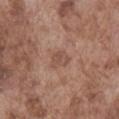Notes:
* follow-up — total-body-photography surveillance lesion; no biopsy
* size — about 2.5 mm
* lighting — white-light illumination
* anatomic site — the abdomen
* imaging modality — 15 mm crop, total-body photography
* patient — male, aged around 75